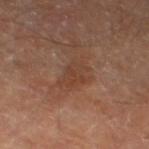Acquisition and patient details: Approximately 4 mm at its widest. The lesion-visualizer software estimated about 5 CIELAB-L* units darker than the surrounding skin and a lesion-to-skin contrast of about 5 (normalized; higher = more distinct). And it measured a border-irregularity index near 4.5/10 and a peripheral color-asymmetry measure near 0.5. The software also gave an automated nevus-likeness rating near 0 out of 100 and a lesion-detection confidence of about 100/100. Imaged with cross-polarized lighting. The lesion is on the left thigh. A close-up tile cropped from a whole-body skin photograph, about 15 mm across. The subject is a male in their 70s.